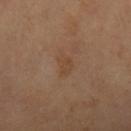- acquisition: 15 mm crop, total-body photography
- subject: female, in their mid-50s
- tile lighting: cross-polarized
- lesion diameter: about 3 mm
- site: the right thigh